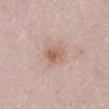* notes — total-body-photography surveillance lesion; no biopsy
* image — total-body-photography crop, ~15 mm field of view
* subject — female, aged 48–52
* automated metrics — a lesion area of about 5 mm² and an eccentricity of roughly 0.55
* lesion size — ~3 mm (longest diameter)
* body site — the abdomen
* lighting — white-light